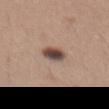Part of a total-body skin-imaging series; this lesion was reviewed on a skin check and was not flagged for biopsy.
A female patient aged around 30.
Captured under white-light illumination.
On the abdomen.
Approximately 3 mm at its widest.
A region of skin cropped from a whole-body photographic capture, roughly 15 mm wide.
Automated tile analysis of the lesion measured a lesion area of about 5.5 mm² and two-axis asymmetry of about 0.2. The analysis additionally found a lesion color around L≈46 a*≈16 b*≈21 in CIELAB and about 17 CIELAB-L* units darker than the surrounding skin. The analysis additionally found a lesion-detection confidence of about 100/100.